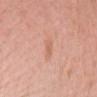Impression:
Captured during whole-body skin photography for melanoma surveillance; the lesion was not biopsied.
Acquisition and patient details:
The lesion's longest dimension is about 3 mm. A female subject in their mid-60s. A roughly 15 mm field-of-view crop from a total-body skin photograph. Imaged with white-light lighting. From the chest.